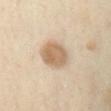Acquisition and patient details: A 15 mm close-up extracted from a 3D total-body photography capture. A female patient about 40 years old. Automated image analysis of the tile measured an area of roughly 12 mm², an eccentricity of roughly 0.55, and a symmetry-axis asymmetry near 0.15. It also reported a normalized lesion–skin contrast near 8. The lesion is located on the chest. Approximately 4 mm at its widest. The tile uses cross-polarized illumination.